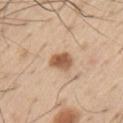follow-up: catalogued during a skin exam; not biopsied
image source: ~15 mm crop, total-body skin-cancer survey
patient: male, aged 53–57
site: the left upper arm